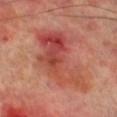| feature | finding |
|---|---|
| biopsy status | total-body-photography surveillance lesion; no biopsy |
| location | the right lower leg |
| subject | male, aged 68 to 72 |
| acquisition | ~15 mm tile from a whole-body skin photo |
| TBP lesion metrics | an average lesion color of about L≈48 a*≈31 b*≈30 (CIELAB) and a lesion-to-skin contrast of about 7 (normalized; higher = more distinct); a nevus-likeness score of about 0/100 and a detector confidence of about 100 out of 100 that the crop contains a lesion |